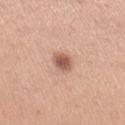A female subject, roughly 30 years of age.
Measured at roughly 2.5 mm in maximum diameter.
The lesion is on the arm.
Captured under white-light illumination.
A 15 mm close-up extracted from a 3D total-body photography capture.